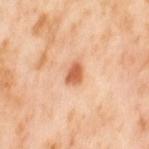| key | value |
|---|---|
| notes | imaged on a skin check; not biopsied |
| image-analysis metrics | a mean CIELAB color near L≈63 a*≈28 b*≈39, about 15 CIELAB-L* units darker than the surrounding skin, and a normalized border contrast of about 9; an automated nevus-likeness rating near 95 out of 100 |
| image | 15 mm crop, total-body photography |
| lesion size | about 2.5 mm |
| anatomic site | the left thigh |
| patient | female, in their mid- to late 50s |
| tile lighting | cross-polarized illumination |